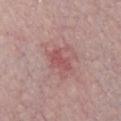  image:
    source: total-body photography crop
    field_of_view_mm: 15
  lesion_size:
    long_diameter_mm_approx: 3.0
  patient:
    sex: male
    age_approx: 30
  lighting: white-light